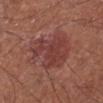The lesion was tiled from a total-body skin photograph and was not biopsied. Imaged with white-light lighting. A region of skin cropped from a whole-body photographic capture, roughly 15 mm wide. On the left lower leg. A male subject, approximately 70 years of age.